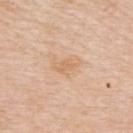follow-up = imaged on a skin check; not biopsied
acquisition = total-body-photography crop, ~15 mm field of view
illumination = white-light illumination
location = the upper back
diameter = ≈3.5 mm
subject = male, aged approximately 75